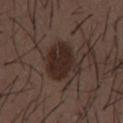Impression:
Recorded during total-body skin imaging; not selected for excision or biopsy.
Context:
A male patient, aged approximately 50. From the mid back. A close-up tile cropped from a whole-body skin photograph, about 15 mm across.